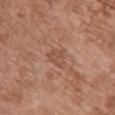Q: Is there a histopathology result?
A: total-body-photography surveillance lesion; no biopsy
Q: How large is the lesion?
A: ~2.5 mm (longest diameter)
Q: What are the patient's age and sex?
A: female, in their mid- to late 70s
Q: Illumination type?
A: white-light illumination
Q: How was this image acquired?
A: total-body-photography crop, ~15 mm field of view
Q: Lesion location?
A: the upper back